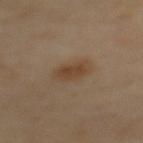Assessment:
The lesion was photographed on a routine skin check and not biopsied; there is no pathology result.
Acquisition and patient details:
This image is a 15 mm lesion crop taken from a total-body photograph. The lesion is located on the mid back. This is a cross-polarized tile. The subject is a male in their mid- to late 60s.